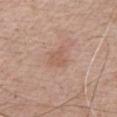workup — total-body-photography surveillance lesion; no biopsy | diameter — about 3 mm | imaging modality — total-body-photography crop, ~15 mm field of view | location — the chest | automated lesion analysis — lesion-presence confidence of about 100/100 | tile lighting — white-light illumination | patient — male, in their mid-60s.